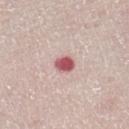No biopsy was performed on this lesion — it was imaged during a full skin examination and was not determined to be concerning. On the left lower leg. A region of skin cropped from a whole-body photographic capture, roughly 15 mm wide. This is a white-light tile. A female patient about 55 years old. The recorded lesion diameter is about 2.5 mm.